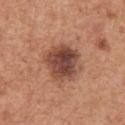Acquisition and patient details: On the chest. Cropped from a total-body skin-imaging series; the visible field is about 15 mm. A male patient, aged around 65. The lesion's longest dimension is about 4.5 mm. The tile uses white-light illumination.